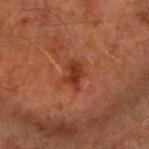workup: total-body-photography surveillance lesion; no biopsy
lighting: cross-polarized illumination
subject: male, aged approximately 60
location: the right forearm
image-analysis metrics: a footprint of about 5 mm²; an average lesion color of about L≈30 a*≈25 b*≈28 (CIELAB), about 9 CIELAB-L* units darker than the surrounding skin, and a lesion-to-skin contrast of about 8 (normalized; higher = more distinct); a nevus-likeness score of about 50/100 and a lesion-detection confidence of about 100/100
size: about 3 mm
image: 15 mm crop, total-body photography